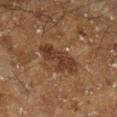* notes · total-body-photography surveillance lesion; no biopsy
* tile lighting · cross-polarized illumination
* site · the right lower leg
* patient · male, roughly 60 years of age
* image · total-body-photography crop, ~15 mm field of view